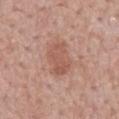{"biopsy_status": "not biopsied; imaged during a skin examination", "lighting": "white-light", "automated_metrics": {"area_mm2_approx": 8.5, "eccentricity": 0.75, "shape_asymmetry": 0.2, "border_irregularity_0_10": 3.0, "color_variation_0_10": 2.5, "peripheral_color_asymmetry": 1.0, "nevus_likeness_0_100": 10, "lesion_detection_confidence_0_100": 100}, "site": "mid back", "lesion_size": {"long_diameter_mm_approx": 4.0}, "patient": {"sex": "male", "age_approx": 50}, "image": {"source": "total-body photography crop", "field_of_view_mm": 15}}